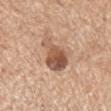No biopsy was performed on this lesion — it was imaged during a full skin examination and was not determined to be concerning. Cropped from a total-body skin-imaging series; the visible field is about 15 mm. A male subject, aged 58 to 62. From the head or neck. An algorithmic analysis of the crop reported two-axis asymmetry of about 0.4. And it measured a lesion color around L≈55 a*≈20 b*≈30 in CIELAB and a lesion-to-skin contrast of about 9 (normalized; higher = more distinct). The analysis additionally found peripheral color asymmetry of about 3. It also reported an automated nevus-likeness rating near 75 out of 100 and lesion-presence confidence of about 100/100. Longest diameter approximately 5.5 mm. The tile uses white-light illumination.